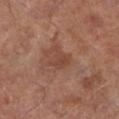Case summary:
* biopsy status — total-body-photography surveillance lesion; no biopsy
* patient — male, approximately 70 years of age
* body site — the left lower leg
* tile lighting — white-light
* image — 15 mm crop, total-body photography